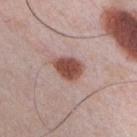Part of a total-body skin-imaging series; this lesion was reviewed on a skin check and was not flagged for biopsy.
A 15 mm close-up tile from a total-body photography series done for melanoma screening.
A male subject, aged 33–37.
The lesion-visualizer software estimated an average lesion color of about L≈49 a*≈22 b*≈25 (CIELAB), roughly 15 lightness units darker than nearby skin, and a normalized border contrast of about 11.
Located on the chest.